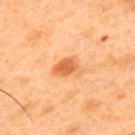biopsy status: catalogued during a skin exam; not biopsied
acquisition: 15 mm crop, total-body photography
TBP lesion metrics: a lesion area of about 6 mm², an eccentricity of roughly 0.75, and a symmetry-axis asymmetry near 0.3; a border-irregularity index near 3/10, internal color variation of about 3 on a 0–10 scale, and a peripheral color-asymmetry measure near 1
patient: male, roughly 50 years of age
body site: the back